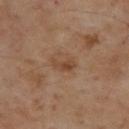Assessment: Part of a total-body skin-imaging series; this lesion was reviewed on a skin check and was not flagged for biopsy. Clinical summary: On the upper back. This image is a 15 mm lesion crop taken from a total-body photograph. The patient is a male in their mid- to late 50s.